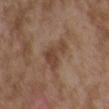Recorded during total-body skin imaging; not selected for excision or biopsy.
This image is a 15 mm lesion crop taken from a total-body photograph.
Automated image analysis of the tile measured an eccentricity of roughly 0.65 and a shape-asymmetry score of about 0.65 (0 = symmetric). The analysis additionally found about 8 CIELAB-L* units darker than the surrounding skin. The software also gave a nevus-likeness score of about 0/100 and a detector confidence of about 100 out of 100 that the crop contains a lesion.
Imaged with white-light lighting.
The recorded lesion diameter is about 4 mm.
A female patient, in their mid-30s.
The lesion is located on the upper back.